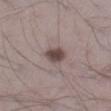Clinical impression:
Imaged during a routine full-body skin examination; the lesion was not biopsied and no histopathology is available.
Context:
The lesion is on the right lower leg. This image is a 15 mm lesion crop taken from a total-body photograph. A male subject, aged 23 to 27. The tile uses white-light illumination.